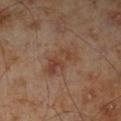<record>
  <biopsy_status>not biopsied; imaged during a skin examination</biopsy_status>
  <image>
    <source>total-body photography crop</source>
    <field_of_view_mm>15</field_of_view_mm>
  </image>
  <patient>
    <sex>male</sex>
    <age_approx>70</age_approx>
  </patient>
  <site>left lower leg</site>
</record>